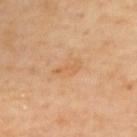Part of a total-body skin-imaging series; this lesion was reviewed on a skin check and was not flagged for biopsy.
The lesion is on the upper back.
Automated tile analysis of the lesion measured a mean CIELAB color near L≈62 a*≈21 b*≈40, a lesion–skin lightness drop of about 6, and a normalized border contrast of about 5. It also reported a border-irregularity rating of about 4.5/10, a color-variation rating of about 0.5/10, and radial color variation of about 0. And it measured a lesion-detection confidence of about 100/100.
A male patient in their mid- to late 60s.
A 15 mm crop from a total-body photograph taken for skin-cancer surveillance.